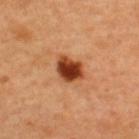| feature | finding |
|---|---|
| follow-up | total-body-photography surveillance lesion; no biopsy |
| image | ~15 mm crop, total-body skin-cancer survey |
| anatomic site | the back |
| patient | male, aged 43 to 47 |